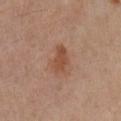biopsy_status: not biopsied; imaged during a skin examination
patient:
  sex: female
  age_approx: 50
image:
  source: total-body photography crop
  field_of_view_mm: 15
site: leg
lesion_size:
  long_diameter_mm_approx: 3.5
lighting: cross-polarized